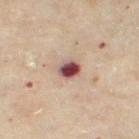Assessment:
The lesion was tiled from a total-body skin photograph and was not biopsied.
Acquisition and patient details:
The lesion is located on the right thigh. A 15 mm close-up tile from a total-body photography series done for melanoma screening. A female subject approximately 60 years of age.